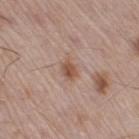The lesion was tiled from a total-body skin photograph and was not biopsied.
A 15 mm close-up tile from a total-body photography series done for melanoma screening.
A male patient about 55 years old.
The lesion is located on the leg.